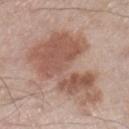Case summary:
- follow-up — no biopsy performed (imaged during a skin exam)
- lesion size — about 11 mm
- tile lighting — white-light illumination
- patient — male, aged 58–62
- body site — the right lower leg
- TBP lesion metrics — a classifier nevus-likeness of about 60/100 and lesion-presence confidence of about 100/100
- image source — total-body-photography crop, ~15 mm field of view